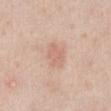Part of a total-body skin-imaging series; this lesion was reviewed on a skin check and was not flagged for biopsy.
A 15 mm close-up tile from a total-body photography series done for melanoma screening.
Located on the abdomen.
The recorded lesion diameter is about 3 mm.
Imaged with white-light lighting.
The subject is a male aged 38 to 42.